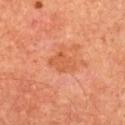A roughly 15 mm field-of-view crop from a total-body skin photograph. A male patient, in their mid-60s. The lesion's longest dimension is about 3.5 mm. From the chest. The tile uses cross-polarized illumination. The total-body-photography lesion software estimated an area of roughly 6 mm², an eccentricity of roughly 0.75, and a shape-asymmetry score of about 0.2 (0 = symmetric). It also reported an automated nevus-likeness rating near 0 out of 100 and a detector confidence of about 100 out of 100 that the crop contains a lesion.